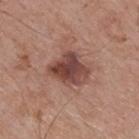Impression: This lesion was catalogued during total-body skin photography and was not selected for biopsy. Clinical summary: The lesion is on the back. A male patient, aged 68 to 72. A lesion tile, about 15 mm wide, cut from a 3D total-body photograph.